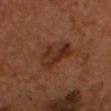Assessment: Imaged during a routine full-body skin examination; the lesion was not biopsied and no histopathology is available. Context: On the head or neck. This is a cross-polarized tile. The total-body-photography lesion software estimated an area of roughly 9 mm². The software also gave about 8 CIELAB-L* units darker than the surrounding skin and a normalized lesion–skin contrast near 8. And it measured a border-irregularity index near 5.5/10, internal color variation of about 4 on a 0–10 scale, and radial color variation of about 1.5. It also reported a classifier nevus-likeness of about 5/100. A lesion tile, about 15 mm wide, cut from a 3D total-body photograph. A male subject aged 48–52. Measured at roughly 4 mm in maximum diameter.